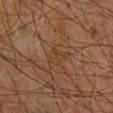The lesion was tiled from a total-body skin photograph and was not biopsied. The patient is a male in their 70s. Cropped from a total-body skin-imaging series; the visible field is about 15 mm. Automated tile analysis of the lesion measured a footprint of about 4.5 mm² and a shape-asymmetry score of about 0.35 (0 = symmetric). The software also gave border irregularity of about 3.5 on a 0–10 scale and radial color variation of about 0.5. This is a cross-polarized tile. From the right forearm.